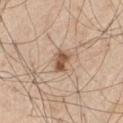workup = catalogued during a skin exam; not biopsied | lesion size = ≈2.5 mm | anatomic site = the left thigh | patient = male, aged approximately 70 | imaging modality = ~15 mm crop, total-body skin-cancer survey | lighting = white-light illumination.